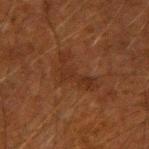Recorded during total-body skin imaging; not selected for excision or biopsy. About 5 mm across. A male subject roughly 50 years of age. On the right upper arm. Cropped from a whole-body photographic skin survey; the tile spans about 15 mm.